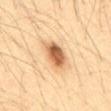Impression:
Captured during whole-body skin photography for melanoma surveillance; the lesion was not biopsied.
Acquisition and patient details:
Longest diameter approximately 4 mm. A male patient aged 33 to 37. The tile uses cross-polarized illumination. Cropped from a total-body skin-imaging series; the visible field is about 15 mm. Located on the front of the torso.